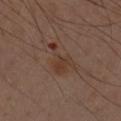notes — imaged on a skin check; not biopsied | acquisition — 15 mm crop, total-body photography | automated metrics — an area of roughly 7 mm², an outline eccentricity of about 0.8 (0 = round, 1 = elongated), and two-axis asymmetry of about 0.75; a lesion color around L≈36 a*≈18 b*≈25 in CIELAB and a lesion–skin lightness drop of about 6; a classifier nevus-likeness of about 0/100 | site — the left forearm | tile lighting — cross-polarized illumination | lesion diameter — ≈4.5 mm | patient — male, about 55 years old.